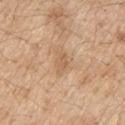Captured during whole-body skin photography for melanoma surveillance; the lesion was not biopsied.
Measured at roughly 2.5 mm in maximum diameter.
Located on the upper back.
This is a white-light tile.
A male patient about 70 years old.
The lesion-visualizer software estimated border irregularity of about 3 on a 0–10 scale, internal color variation of about 2 on a 0–10 scale, and radial color variation of about 1. The software also gave a lesion-detection confidence of about 100/100.
A close-up tile cropped from a whole-body skin photograph, about 15 mm across.